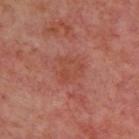Case summary:
- workup · total-body-photography surveillance lesion; no biopsy
- image source · ~15 mm crop, total-body skin-cancer survey
- location · the upper back
- patient · aged around 55